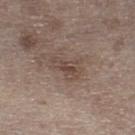Assessment: No biopsy was performed on this lesion — it was imaged during a full skin examination and was not determined to be concerning. Image and clinical context: A region of skin cropped from a whole-body photographic capture, roughly 15 mm wide. A male patient, approximately 70 years of age. Imaged with white-light lighting. The lesion is on the right lower leg.